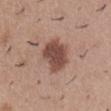{
  "biopsy_status": "not biopsied; imaged during a skin examination",
  "site": "abdomen",
  "image": {
    "source": "total-body photography crop",
    "field_of_view_mm": 15
  },
  "patient": {
    "sex": "male",
    "age_approx": 30
  }
}